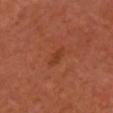Background: A close-up tile cropped from a whole-body skin photograph, about 15 mm across. The lesion's longest dimension is about 3 mm. On the chest. A female subject in their mid-50s. The lesion-visualizer software estimated a border-irregularity rating of about 3/10 and internal color variation of about 1 on a 0–10 scale.